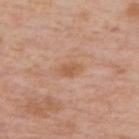Part of a total-body skin-imaging series; this lesion was reviewed on a skin check and was not flagged for biopsy. The patient is a male aged around 75. The lesion is located on the upper back. Cropped from a whole-body photographic skin survey; the tile spans about 15 mm. The lesion's longest dimension is about 2.5 mm.